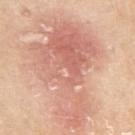workup: no biopsy performed (imaged during a skin exam) | site: the left upper arm | patient: female, aged 68–72 | lesion diameter: about 12.5 mm | image: 15 mm crop, total-body photography | automated metrics: a symmetry-axis asymmetry near 0.5; border irregularity of about 8 on a 0–10 scale, a color-variation rating of about 6.5/10, and peripheral color asymmetry of about 2.5; a lesion-detection confidence of about 100/100 | tile lighting: white-light illumination.